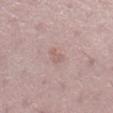workup: imaged on a skin check; not biopsied
subject: female, aged approximately 50
location: the right lower leg
automated lesion analysis: a lesion area of about 3 mm² and a shape-asymmetry score of about 0.45 (0 = symmetric); an average lesion color of about L≈60 a*≈18 b*≈22 (CIELAB) and about 6 CIELAB-L* units darker than the surrounding skin; a border-irregularity index near 4.5/10 and internal color variation of about 0.5 on a 0–10 scale; a nevus-likeness score of about 0/100 and a detector confidence of about 100 out of 100 that the crop contains a lesion
size: about 2.5 mm
imaging modality: 15 mm crop, total-body photography
tile lighting: white-light illumination